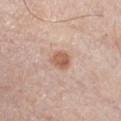The lesion was photographed on a routine skin check and not biopsied; there is no pathology result.
The total-body-photography lesion software estimated an average lesion color of about L≈59 a*≈21 b*≈30 (CIELAB), a lesion–skin lightness drop of about 11, and a lesion-to-skin contrast of about 8 (normalized; higher = more distinct). It also reported an automated nevus-likeness rating near 90 out of 100 and lesion-presence confidence of about 100/100.
A close-up tile cropped from a whole-body skin photograph, about 15 mm across.
About 2.5 mm across.
The subject is a male roughly 70 years of age.
Imaged with white-light lighting.
On the chest.